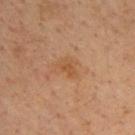Located on the upper back. A close-up tile cropped from a whole-body skin photograph, about 15 mm across. A male subject, aged 33 to 37. Imaged with cross-polarized lighting. Longest diameter approximately 3 mm. Automated image analysis of the tile measured an area of roughly 3.5 mm², a shape eccentricity near 0.75, and two-axis asymmetry of about 0.45. It also reported an average lesion color of about L≈47 a*≈20 b*≈34 (CIELAB), a lesion–skin lightness drop of about 6, and a normalized lesion–skin contrast near 6.